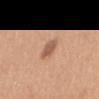Part of a total-body skin-imaging series; this lesion was reviewed on a skin check and was not flagged for biopsy.
An algorithmic analysis of the crop reported a mean CIELAB color near L≈57 a*≈22 b*≈32, a lesion–skin lightness drop of about 11, and a normalized border contrast of about 7.5. The software also gave a border-irregularity rating of about 2/10, internal color variation of about 1.5 on a 0–10 scale, and peripheral color asymmetry of about 0.5.
Imaged with white-light lighting.
A female patient, aged 28 to 32.
Located on the mid back.
Cropped from a total-body skin-imaging series; the visible field is about 15 mm.